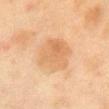| field | value |
|---|---|
| follow-up | imaged on a skin check; not biopsied |
| anatomic site | the chest |
| patient | female, aged approximately 50 |
| acquisition | ~15 mm tile from a whole-body skin photo |